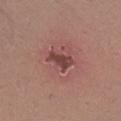No biopsy was performed on this lesion — it was imaged during a full skin examination and was not determined to be concerning.
A roughly 15 mm field-of-view crop from a total-body skin photograph.
Imaged with white-light lighting.
The patient is a female roughly 35 years of age.
The lesion is located on the right lower leg.
About 3.5 mm across.